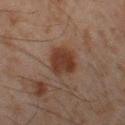Findings:
– follow-up — no biopsy performed (imaged during a skin exam)
– location — the left thigh
– tile lighting — cross-polarized
– patient — male, aged 43–47
– image source — ~15 mm tile from a whole-body skin photo
– diameter — ~4.5 mm (longest diameter)
– automated lesion analysis — a classifier nevus-likeness of about 95/100 and lesion-presence confidence of about 100/100